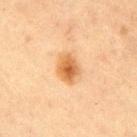  biopsy_status: not biopsied; imaged during a skin examination
  lesion_size:
    long_diameter_mm_approx: 3.5
  patient:
    sex: male
    age_approx: 60
  site: right upper arm
  lighting: cross-polarized
  image:
    source: total-body photography crop
    field_of_view_mm: 15
  automated_metrics:
    vs_skin_darker_L: 11.0
    vs_skin_contrast_norm: 8.5